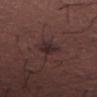notes — total-body-photography surveillance lesion; no biopsy.